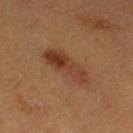Recorded during total-body skin imaging; not selected for excision or biopsy. This image is a 15 mm lesion crop taken from a total-body photograph. A female patient, aged approximately 40. An algorithmic analysis of the crop reported a classifier nevus-likeness of about 75/100 and a detector confidence of about 100 out of 100 that the crop contains a lesion. Located on the leg. Captured under cross-polarized illumination.